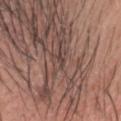Image and clinical context:
On the head or neck. A male subject aged around 55. The recorded lesion diameter is about 2 mm. This image is a 15 mm lesion crop taken from a total-body photograph. Imaged with white-light lighting.